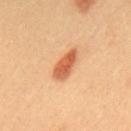notes: imaged on a skin check; not biopsied
acquisition: total-body-photography crop, ~15 mm field of view
subject: female, aged 28–32
anatomic site: the upper back